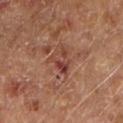  biopsy_status: not biopsied; imaged during a skin examination
  patient:
    sex: male
    age_approx: 65
  image:
    source: total-body photography crop
    field_of_view_mm: 15
  lesion_size:
    long_diameter_mm_approx: 3.5
  site: left upper arm
  automated_metrics:
    area_mm2_approx: 5.0
    eccentricity: 0.7
    border_irregularity_0_10: 4.5
    color_variation_0_10: 10.0
    peripheral_color_asymmetry: 3.5
    nevus_likeness_0_100: 0
    lesion_detection_confidence_0_100: 100